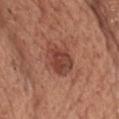Impression:
Captured during whole-body skin photography for melanoma surveillance; the lesion was not biopsied.
Image and clinical context:
The lesion is located on the chest. About 5 mm across. This is a white-light tile. The total-body-photography lesion software estimated a mean CIELAB color near L≈43 a*≈25 b*≈28 and roughly 10 lightness units darker than nearby skin. The analysis additionally found a classifier nevus-likeness of about 65/100 and lesion-presence confidence of about 100/100. A male subject roughly 60 years of age. Cropped from a total-body skin-imaging series; the visible field is about 15 mm.